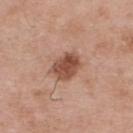<record>
<biopsy_status>not biopsied; imaged during a skin examination</biopsy_status>
<lesion_size>
  <long_diameter_mm_approx>4.0</long_diameter_mm_approx>
</lesion_size>
<image>
  <source>total-body photography crop</source>
  <field_of_view_mm>15</field_of_view_mm>
</image>
<patient>
  <sex>male</sex>
  <age_approx>65</age_approx>
</patient>
<site>upper back</site>
</record>